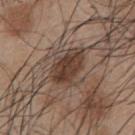Assessment: The lesion was tiled from a total-body skin photograph and was not biopsied. Image and clinical context: The subject is a male approximately 45 years of age. The lesion is located on the chest. Cropped from a whole-body photographic skin survey; the tile spans about 15 mm.